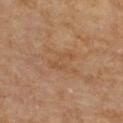Findings:
* follow-up — imaged on a skin check; not biopsied
* patient — male, roughly 85 years of age or older
* site — the upper back
* lesion diameter — ≈3.5 mm
* TBP lesion metrics — an area of roughly 4 mm², an eccentricity of roughly 0.9, and a shape-asymmetry score of about 0.5 (0 = symmetric); a lesion color around L≈49 a*≈19 b*≈34 in CIELAB and a normalized border contrast of about 4.5; a border-irregularity rating of about 6.5/10, a within-lesion color-variation index near 1/10, and a peripheral color-asymmetry measure near 0; a classifier nevus-likeness of about 0/100 and lesion-presence confidence of about 100/100
* imaging modality — ~15 mm tile from a whole-body skin photo